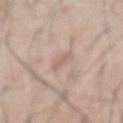  patient:
    sex: male
    age_approx: 60
  image:
    source: total-body photography crop
    field_of_view_mm: 15
  site: abdomen